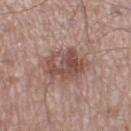Captured during whole-body skin photography for melanoma surveillance; the lesion was not biopsied. A male patient approximately 55 years of age. The lesion is located on the left thigh. A close-up tile cropped from a whole-body skin photograph, about 15 mm across. Approximately 5 mm at its widest. The total-body-photography lesion software estimated an average lesion color of about L≈50 a*≈19 b*≈23 (CIELAB), about 10 CIELAB-L* units darker than the surrounding skin, and a normalized border contrast of about 7.5. It also reported border irregularity of about 3 on a 0–10 scale. The software also gave a detector confidence of about 100 out of 100 that the crop contains a lesion. Imaged with white-light lighting.